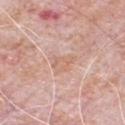Background: A 15 mm close-up tile from a total-body photography series done for melanoma screening. Located on the chest. The patient is a male aged 78 to 82.